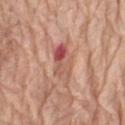Q: Was a biopsy performed?
A: catalogued during a skin exam; not biopsied
Q: What is the anatomic site?
A: the right thigh
Q: What is the imaging modality?
A: total-body-photography crop, ~15 mm field of view
Q: How was the tile lit?
A: white-light illumination
Q: Who is the patient?
A: female, in their mid- to late 70s
Q: Automated lesion metrics?
A: an outline eccentricity of about 0.85 (0 = round, 1 = elongated) and two-axis asymmetry of about 0.2; a mean CIELAB color near L≈55 a*≈26 b*≈27, about 10 CIELAB-L* units darker than the surrounding skin, and a lesion-to-skin contrast of about 7 (normalized; higher = more distinct)